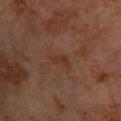Q: Is there a histopathology result?
A: no biopsy performed (imaged during a skin exam)
Q: Illumination type?
A: cross-polarized illumination
Q: Lesion location?
A: the upper back
Q: What are the patient's age and sex?
A: male, approximately 70 years of age
Q: Lesion size?
A: about 2.5 mm
Q: How was this image acquired?
A: ~15 mm crop, total-body skin-cancer survey